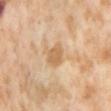Q: Was a biopsy performed?
A: catalogued during a skin exam; not biopsied
Q: How was this image acquired?
A: ~15 mm tile from a whole-body skin photo
Q: What did automated image analysis measure?
A: a footprint of about 6 mm², a shape eccentricity near 0.7, and two-axis asymmetry of about 0.3; border irregularity of about 3 on a 0–10 scale and a peripheral color-asymmetry measure near 0.5; a lesion-detection confidence of about 100/100
Q: Patient demographics?
A: female, about 55 years old
Q: Lesion location?
A: the leg
Q: Illumination type?
A: cross-polarized illumination
Q: What is the lesion's diameter?
A: about 3.5 mm